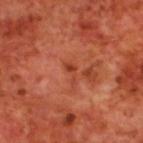follow-up=imaged on a skin check; not biopsied | subject=male, aged approximately 70 | lesion size=≈2.5 mm | tile lighting=cross-polarized | body site=the upper back | acquisition=total-body-photography crop, ~15 mm field of view.